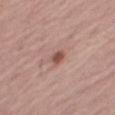The lesion was photographed on a routine skin check and not biopsied; there is no pathology result.
The recorded lesion diameter is about 2 mm.
The patient is a female roughly 65 years of age.
The tile uses white-light illumination.
On the left leg.
A region of skin cropped from a whole-body photographic capture, roughly 15 mm wide.